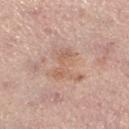Findings:
* follow-up — total-body-photography surveillance lesion; no biopsy
* subject — female, roughly 65 years of age
* location — the leg
* imaging modality — ~15 mm crop, total-body skin-cancer survey